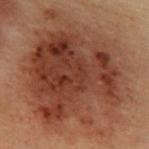Part of a total-body skin-imaging series; this lesion was reviewed on a skin check and was not flagged for biopsy. Measured at roughly 15 mm in maximum diameter. A male subject approximately 55 years of age. The lesion is on the abdomen. Captured under cross-polarized illumination. The total-body-photography lesion software estimated a lesion color around L≈33 a*≈19 b*≈25 in CIELAB and about 10 CIELAB-L* units darker than the surrounding skin. The analysis additionally found border irregularity of about 3.5 on a 0–10 scale, internal color variation of about 7 on a 0–10 scale, and peripheral color asymmetry of about 2.5. And it measured a nevus-likeness score of about 20/100. A close-up tile cropped from a whole-body skin photograph, about 15 mm across.